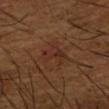<record>
<biopsy_status>not biopsied; imaged during a skin examination</biopsy_status>
<patient>
  <sex>male</sex>
  <age_approx>65</age_approx>
</patient>
<automated_metrics>
  <cielab_L>29</cielab_L>
  <cielab_a>21</cielab_a>
  <cielab_b>26</cielab_b>
  <vs_skin_darker_L>5.0</vs_skin_darker_L>
  <vs_skin_contrast_norm>5.5</vs_skin_contrast_norm>
  <border_irregularity_0_10>5.0</border_irregularity_0_10>
  <color_variation_0_10>4.5</color_variation_0_10>
  <peripheral_color_asymmetry>1.5</peripheral_color_asymmetry>
  <nevus_likeness_0_100>0</nevus_likeness_0_100>
  <lesion_detection_confidence_0_100>100</lesion_detection_confidence_0_100>
</automated_metrics>
<image>
  <source>total-body photography crop</source>
  <field_of_view_mm>15</field_of_view_mm>
</image>
<site>right forearm</site>
<lighting>cross-polarized</lighting>
</record>